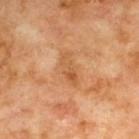This lesion was catalogued during total-body skin photography and was not selected for biopsy.
The lesion-visualizer software estimated an area of roughly 5.5 mm² and two-axis asymmetry of about 0.3. The software also gave an average lesion color of about L≈55 a*≈23 b*≈40 (CIELAB) and roughly 8 lightness units darker than nearby skin.
A 15 mm close-up extracted from a 3D total-body photography capture.
Located on the upper back.
The subject is a male about 70 years old.
The tile uses cross-polarized illumination.
The recorded lesion diameter is about 4 mm.